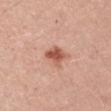Q: Is there a histopathology result?
A: no biopsy performed (imaged during a skin exam)
Q: Automated lesion metrics?
A: a mean CIELAB color near L≈56 a*≈27 b*≈31 and roughly 13 lightness units darker than nearby skin; a detector confidence of about 100 out of 100 that the crop contains a lesion
Q: What is the anatomic site?
A: the left upper arm
Q: How was this image acquired?
A: total-body-photography crop, ~15 mm field of view
Q: Lesion size?
A: about 3 mm
Q: Who is the patient?
A: male, aged approximately 40